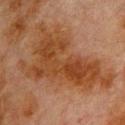Imaged during a routine full-body skin examination; the lesion was not biopsied and no histopathology is available.
A male subject, aged 78–82.
Cropped from a whole-body photographic skin survey; the tile spans about 15 mm.
The lesion is located on the upper back.